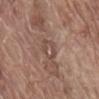Q: Was a biopsy performed?
A: imaged on a skin check; not biopsied
Q: What is the imaging modality?
A: total-body-photography crop, ~15 mm field of view
Q: Where on the body is the lesion?
A: the front of the torso
Q: Automated lesion metrics?
A: a lesion-detection confidence of about 75/100
Q: Who is the patient?
A: male, roughly 80 years of age